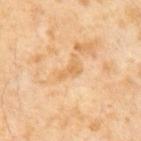{"biopsy_status": "not biopsied; imaged during a skin examination"}